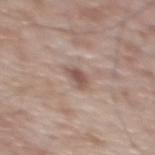Clinical summary:
The recorded lesion diameter is about 2.5 mm. A lesion tile, about 15 mm wide, cut from a 3D total-body photograph. On the back. Automated image analysis of the tile measured a mean CIELAB color near L≈53 a*≈17 b*≈23, roughly 11 lightness units darker than nearby skin, and a normalized lesion–skin contrast near 7.5. The analysis additionally found a border-irregularity rating of about 2.5/10, a color-variation rating of about 1.5/10, and peripheral color asymmetry of about 0.5. The analysis additionally found a nevus-likeness score of about 50/100 and a lesion-detection confidence of about 100/100. The patient is a male aged 68 to 72. Captured under white-light illumination.